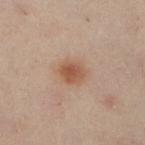biopsy status = no biopsy performed (imaged during a skin exam) | site = the right lower leg | acquisition = ~15 mm crop, total-body skin-cancer survey | patient = female, in their mid- to late 50s | illumination = cross-polarized | size = ≈3 mm.